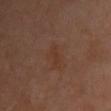workup = no biopsy performed (imaged during a skin exam).